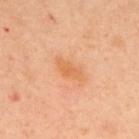Impression: Imaged during a routine full-body skin examination; the lesion was not biopsied and no histopathology is available. Background: The lesion is on the upper back. A 15 mm close-up extracted from a 3D total-body photography capture. Automated image analysis of the tile measured a footprint of about 4.5 mm², a shape eccentricity near 0.9, and a symmetry-axis asymmetry near 0.4. The analysis additionally found an average lesion color of about L≈64 a*≈25 b*≈42 (CIELAB) and roughly 7 lightness units darker than nearby skin. A male subject in their 40s. The tile uses cross-polarized illumination.